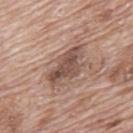biopsy status=catalogued during a skin exam; not biopsied
illumination=white-light illumination
TBP lesion metrics=border irregularity of about 4 on a 0–10 scale, internal color variation of about 4.5 on a 0–10 scale, and radial color variation of about 2; a nevus-likeness score of about 5/100 and a lesion-detection confidence of about 100/100
patient=male, aged approximately 70
imaging modality=~15 mm crop, total-body skin-cancer survey
location=the back
size=about 5.5 mm